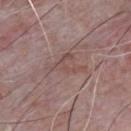This lesion was catalogued during total-body skin photography and was not selected for biopsy.
Measured at roughly 5 mm in maximum diameter.
A male patient about 65 years old.
Imaged with white-light lighting.
This image is a 15 mm lesion crop taken from a total-body photograph.
Located on the chest.